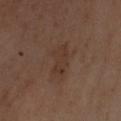lighting: cross-polarized | size: about 4 mm | location: the left forearm | TBP lesion metrics: a mean CIELAB color near L≈35 a*≈17 b*≈25 and a lesion–skin lightness drop of about 5; a lesion-detection confidence of about 100/100 | patient: female, in their mid-50s | image: 15 mm crop, total-body photography.